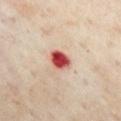Impression: The lesion was photographed on a routine skin check and not biopsied; there is no pathology result. Clinical summary: Captured under cross-polarized illumination. Located on the chest. A female patient approximately 60 years of age. A roughly 15 mm field-of-view crop from a total-body skin photograph. The recorded lesion diameter is about 3 mm.